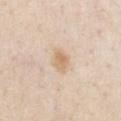{"biopsy_status": "not biopsied; imaged during a skin examination", "automated_metrics": {"border_irregularity_0_10": 2.5, "color_variation_0_10": 2.5}, "patient": {"sex": "male", "age_approx": 45}, "site": "chest", "image": {"source": "total-body photography crop", "field_of_view_mm": 15}}